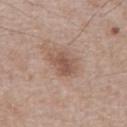Imaged during a routine full-body skin examination; the lesion was not biopsied and no histopathology is available. A roughly 15 mm field-of-view crop from a total-body skin photograph. On the abdomen. A male patient, aged approximately 50.